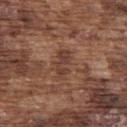From the upper back. This is a white-light tile. An algorithmic analysis of the crop reported a shape-asymmetry score of about 0.3 (0 = symmetric). And it measured radial color variation of about 0.5. It also reported an automated nevus-likeness rating near 0 out of 100. Approximately 3.5 mm at its widest. A close-up tile cropped from a whole-body skin photograph, about 15 mm across. A male subject in their mid- to late 70s.